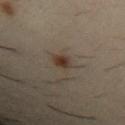Clinical impression: Imaged during a routine full-body skin examination; the lesion was not biopsied and no histopathology is available. Image and clinical context: Automated tile analysis of the lesion measured an area of roughly 4 mm² and a shape-asymmetry score of about 0.15 (0 = symmetric). The analysis additionally found a within-lesion color-variation index near 3/10 and radial color variation of about 1. The analysis additionally found a classifier nevus-likeness of about 95/100 and lesion-presence confidence of about 100/100. A 15 mm close-up tile from a total-body photography series done for melanoma screening. Measured at roughly 3 mm in maximum diameter. A male patient aged around 30. On the left upper arm. Captured under cross-polarized illumination.